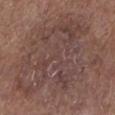<case>
  <biopsy_status>not biopsied; imaged during a skin examination</biopsy_status>
  <automated_metrics>
    <color_variation_0_10>5.5</color_variation_0_10>
    <nevus_likeness_0_100>0</nevus_likeness_0_100>
    <lesion_detection_confidence_0_100>100</lesion_detection_confidence_0_100>
  </automated_metrics>
  <patient>
    <sex>male</sex>
    <age_approx>75</age_approx>
  </patient>
  <site>left lower leg</site>
  <lighting>white-light</lighting>
  <image>
    <source>total-body photography crop</source>
    <field_of_view_mm>15</field_of_view_mm>
  </image>
</case>